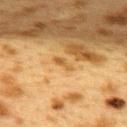workup = no biopsy performed (imaged during a skin exam)
subject = female, aged 38–42
tile lighting = cross-polarized
acquisition = 15 mm crop, total-body photography
anatomic site = the upper back
lesion diameter = ≈2.5 mm
TBP lesion metrics = a lesion color around L≈49 a*≈18 b*≈40 in CIELAB, about 7 CIELAB-L* units darker than the surrounding skin, and a normalized border contrast of about 6; a border-irregularity index near 3/10, a within-lesion color-variation index near 0/10, and radial color variation of about 0; an automated nevus-likeness rating near 0 out of 100 and a detector confidence of about 100 out of 100 that the crop contains a lesion